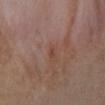workup: catalogued during a skin exam; not biopsied | lesion size: ~1 mm (longest diameter) | body site: the left upper arm | lighting: white-light | patient: female, in their 30s | image: 15 mm crop, total-body photography.